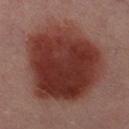Imaged during a routine full-body skin examination; the lesion was not biopsied and no histopathology is available.
An algorithmic analysis of the crop reported an area of roughly 70 mm², an outline eccentricity of about 0.55 (0 = round, 1 = elongated), and two-axis asymmetry of about 0.2.
A female patient aged around 50.
Longest diameter approximately 10.5 mm.
A roughly 15 mm field-of-view crop from a total-body skin photograph.
The lesion is located on the left thigh.
The tile uses cross-polarized illumination.